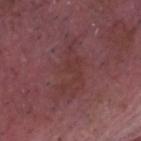This lesion was catalogued during total-body skin photography and was not selected for biopsy. An algorithmic analysis of the crop reported a footprint of about 16 mm², an outline eccentricity of about 0.85 (0 = round, 1 = elongated), and a symmetry-axis asymmetry near 0.45. It also reported a mean CIELAB color near L≈36 a*≈23 b*≈19, about 5 CIELAB-L* units darker than the surrounding skin, and a normalized lesion–skin contrast near 5. The lesion is on the head or neck. The lesion's longest dimension is about 7 mm. A 15 mm close-up extracted from a 3D total-body photography capture. Imaged with white-light lighting. A male patient, aged around 65.